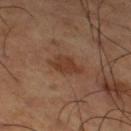Clinical impression:
The lesion was tiled from a total-body skin photograph and was not biopsied.
Acquisition and patient details:
The lesion's longest dimension is about 3.5 mm. Captured under cross-polarized illumination. A male subject, approximately 65 years of age. A roughly 15 mm field-of-view crop from a total-body skin photograph. Located on the right thigh.